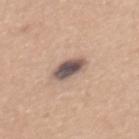| feature | finding |
|---|---|
| follow-up | no biopsy performed (imaged during a skin exam) |
| diameter | ≈3.5 mm |
| acquisition | 15 mm crop, total-body photography |
| anatomic site | the upper back |
| patient | female, aged 28–32 |